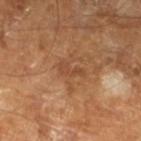- follow-up — total-body-photography surveillance lesion; no biopsy
- patient — male, aged 63 to 67
- illumination — cross-polarized illumination
- image-analysis metrics — an average lesion color of about L≈45 a*≈22 b*≈33 (CIELAB), a lesion–skin lightness drop of about 7, and a lesion-to-skin contrast of about 5.5 (normalized; higher = more distinct); a classifier nevus-likeness of about 0/100 and a lesion-detection confidence of about 100/100
- imaging modality — ~15 mm crop, total-body skin-cancer survey
- size — ~3.5 mm (longest diameter)